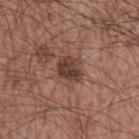Part of a total-body skin-imaging series; this lesion was reviewed on a skin check and was not flagged for biopsy.